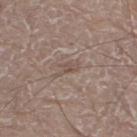Background:
The lesion is on the right leg. Automated image analysis of the tile measured a lesion area of about 3 mm². The analysis additionally found a border-irregularity rating of about 5/10 and radial color variation of about 0.5. It also reported a classifier nevus-likeness of about 0/100 and a detector confidence of about 80 out of 100 that the crop contains a lesion. A male patient, in their 80s. Captured under white-light illumination. A 15 mm close-up extracted from a 3D total-body photography capture. About 2.5 mm across.